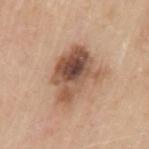The patient is a male aged around 80. A region of skin cropped from a whole-body photographic capture, roughly 15 mm wide. The tile uses white-light illumination. The lesion is on the arm. Approximately 6 mm at its widest. Histopathological examination showed a melanoma in situ — a malignancy.